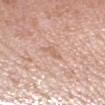Assessment: The lesion was tiled from a total-body skin photograph and was not biopsied. Clinical summary: From the left forearm. The patient is a female approximately 60 years of age. A lesion tile, about 15 mm wide, cut from a 3D total-body photograph.